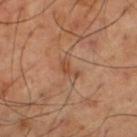Acquisition and patient details:
A 15 mm close-up extracted from a 3D total-body photography capture. The total-body-photography lesion software estimated an eccentricity of roughly 0.85 and a shape-asymmetry score of about 0.6 (0 = symmetric). The analysis additionally found a border-irregularity index near 6/10, a color-variation rating of about 0/10, and radial color variation of about 0. The software also gave a classifier nevus-likeness of about 0/100 and a detector confidence of about 100 out of 100 that the crop contains a lesion. The lesion's longest dimension is about 2.5 mm. A male patient, about 65 years old. From the left thigh.